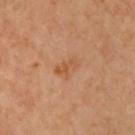TBP lesion metrics: a shape eccentricity near 0.8 and a shape-asymmetry score of about 0.3 (0 = symmetric); a mean CIELAB color near L≈47 a*≈20 b*≈33, about 6 CIELAB-L* units darker than the surrounding skin, and a normalized lesion–skin contrast near 5.5; a nevus-likeness score of about 0/100 and a detector confidence of about 100 out of 100 that the crop contains a lesion
location: the chest
patient: female, aged approximately 60
acquisition: 15 mm crop, total-body photography